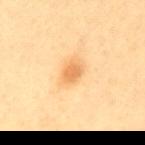Clinical impression: Part of a total-body skin-imaging series; this lesion was reviewed on a skin check and was not flagged for biopsy. Context: On the mid back. The lesion's longest dimension is about 3 mm. A female subject, approximately 45 years of age. This is a cross-polarized tile. A 15 mm crop from a total-body photograph taken for skin-cancer surveillance.